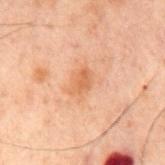Q: What did automated image analysis measure?
A: a lesion area of about 4.5 mm² and a shape eccentricity near 0.8; a mean CIELAB color near L≈49 a*≈20 b*≈31, roughly 7 lightness units darker than nearby skin, and a lesion-to-skin contrast of about 6 (normalized; higher = more distinct); border irregularity of about 3 on a 0–10 scale, internal color variation of about 1.5 on a 0–10 scale, and radial color variation of about 0.5
Q: How was this image acquired?
A: total-body-photography crop, ~15 mm field of view
Q: Where on the body is the lesion?
A: the mid back
Q: What is the lesion's diameter?
A: ~3 mm (longest diameter)
Q: How was the tile lit?
A: cross-polarized illumination
Q: What are the patient's age and sex?
A: male, roughly 60 years of age